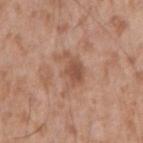The lesion was tiled from a total-body skin photograph and was not biopsied.